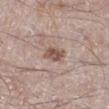Clinical impression: Captured during whole-body skin photography for melanoma surveillance; the lesion was not biopsied. Background: The lesion's longest dimension is about 3 mm. A male patient aged 58 to 62. Cropped from a total-body skin-imaging series; the visible field is about 15 mm. Located on the right lower leg.